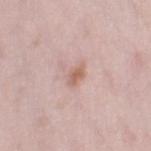{"biopsy_status": "not biopsied; imaged during a skin examination", "image": {"source": "total-body photography crop", "field_of_view_mm": 15}, "site": "right thigh", "lighting": "white-light", "lesion_size": {"long_diameter_mm_approx": 2.5}, "automated_metrics": {"area_mm2_approx": 3.5, "eccentricity": 0.75, "shape_asymmetry": 0.2, "border_irregularity_0_10": 2.0, "color_variation_0_10": 2.5}, "patient": {"sex": "female", "age_approx": 30}}